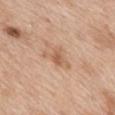The lesion was photographed on a routine skin check and not biopsied; there is no pathology result. The recorded lesion diameter is about 4 mm. This image is a 15 mm lesion crop taken from a total-body photograph. On the mid back. A female subject, approximately 40 years of age. Imaged with white-light lighting.